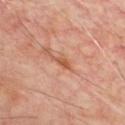{
  "biopsy_status": "not biopsied; imaged during a skin examination",
  "image": {
    "source": "total-body photography crop",
    "field_of_view_mm": 15
  },
  "site": "chest",
  "lighting": "cross-polarized",
  "patient": {
    "sex": "male",
    "age_approx": 65
  }
}